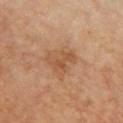Imaged with cross-polarized lighting.
Automated tile analysis of the lesion measured a lesion area of about 5.5 mm², a shape eccentricity near 0.8, and two-axis asymmetry of about 0.45. It also reported radial color variation of about 0.5. And it measured a nevus-likeness score of about 0/100 and lesion-presence confidence of about 100/100.
The lesion is located on the chest.
Cropped from a total-body skin-imaging series; the visible field is about 15 mm.
The subject is a female in their mid- to late 50s.